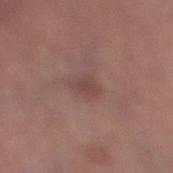<case>
  <biopsy_status>not biopsied; imaged during a skin examination</biopsy_status>
  <lesion_size>
    <long_diameter_mm_approx>3.0</long_diameter_mm_approx>
  </lesion_size>
  <site>right lower leg</site>
  <lighting>white-light</lighting>
  <image>
    <source>total-body photography crop</source>
    <field_of_view_mm>15</field_of_view_mm>
  </image>
  <patient>
    <sex>female</sex>
    <age_approx>60</age_approx>
  </patient>
</case>